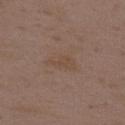The lesion was photographed on a routine skin check and not biopsied; there is no pathology result. This image is a 15 mm lesion crop taken from a total-body photograph. The recorded lesion diameter is about 3.5 mm. Imaged with white-light lighting. Located on the back. The patient is a female aged around 35.